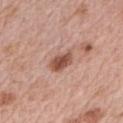notes: imaged on a skin check; not biopsied
automated metrics: a footprint of about 5.5 mm² and an outline eccentricity of about 0.8 (0 = round, 1 = elongated); a mean CIELAB color near L≈52 a*≈23 b*≈28; a border-irregularity rating of about 3/10 and internal color variation of about 3.5 on a 0–10 scale; a detector confidence of about 100 out of 100 that the crop contains a lesion
lesion diameter: ~3 mm (longest diameter)
acquisition: 15 mm crop, total-body photography
illumination: white-light illumination
site: the right upper arm
patient: female, aged approximately 65